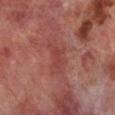follow-up — imaged on a skin check; not biopsied | body site — the right lower leg | lighting — cross-polarized | imaging modality — total-body-photography crop, ~15 mm field of view | patient — male, roughly 70 years of age | diameter — about 3 mm | automated lesion analysis — a lesion area of about 3.5 mm² and an eccentricity of roughly 0.85; a lesion color around L≈41 a*≈28 b*≈25 in CIELAB, roughly 6 lightness units darker than nearby skin, and a normalized lesion–skin contrast near 5; an automated nevus-likeness rating near 0 out of 100 and a detector confidence of about 95 out of 100 that the crop contains a lesion.